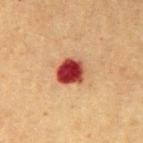Clinical impression: Recorded during total-body skin imaging; not selected for excision or biopsy. Image and clinical context: The lesion is on the right upper arm. Measured at roughly 3.5 mm in maximum diameter. A male patient, roughly 60 years of age. Automated tile analysis of the lesion measured a mean CIELAB color near L≈40 a*≈34 b*≈29, about 21 CIELAB-L* units darker than the surrounding skin, and a normalized border contrast of about 15.5. A roughly 15 mm field-of-view crop from a total-body skin photograph. Captured under cross-polarized illumination.